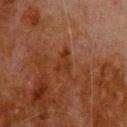A region of skin cropped from a whole-body photographic capture, roughly 15 mm wide.
A male patient roughly 80 years of age.
On the upper back.
Longest diameter approximately 3.5 mm.
Captured under cross-polarized illumination.
An algorithmic analysis of the crop reported an area of roughly 4 mm², an eccentricity of roughly 0.8, and two-axis asymmetry of about 0.6. The analysis additionally found a lesion–skin lightness drop of about 5 and a lesion-to-skin contrast of about 6.5 (normalized; higher = more distinct).